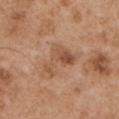notes: total-body-photography surveillance lesion; no biopsy | anatomic site: the left upper arm | size: ~5 mm (longest diameter) | acquisition: ~15 mm crop, total-body skin-cancer survey | illumination: white-light | automated metrics: a lesion area of about 9 mm², an outline eccentricity of about 0.75 (0 = round, 1 = elongated), and a symmetry-axis asymmetry near 0.65 | patient: male, aged 53–57.